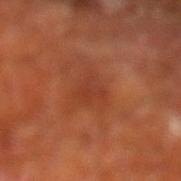Findings:
- follow-up: no biopsy performed (imaged during a skin exam)
- patient: male, aged approximately 65
- image source: total-body-photography crop, ~15 mm field of view
- body site: the left lower leg